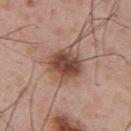Findings:
* notes: no biopsy performed (imaged during a skin exam)
* imaging modality: ~15 mm tile from a whole-body skin photo
* size: about 4.5 mm
* lighting: white-light
* TBP lesion metrics: an average lesion color of about L≈48 a*≈20 b*≈27 (CIELAB) and a lesion-to-skin contrast of about 9.5 (normalized; higher = more distinct)
* patient: male, aged 53 to 57
* body site: the upper back